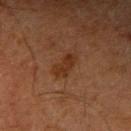biopsy_status: not biopsied; imaged during a skin examination
image:
  source: total-body photography crop
  field_of_view_mm: 15
lesion_size:
  long_diameter_mm_approx: 3.5
site: right upper arm
patient:
  sex: male
  age_approx: 65
lighting: cross-polarized